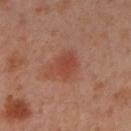Assessment: Part of a total-body skin-imaging series; this lesion was reviewed on a skin check and was not flagged for biopsy. Context: The patient is a female aged approximately 40. An algorithmic analysis of the crop reported a lesion area of about 7 mm², an outline eccentricity of about 0.6 (0 = round, 1 = elongated), and a shape-asymmetry score of about 0.25 (0 = symmetric). And it measured roughly 8 lightness units darker than nearby skin. The software also gave internal color variation of about 2 on a 0–10 scale and peripheral color asymmetry of about 0.5. The software also gave a nevus-likeness score of about 85/100 and a lesion-detection confidence of about 100/100. The lesion is on the arm. Approximately 3.5 mm at its widest. A 15 mm close-up tile from a total-body photography series done for melanoma screening. The tile uses cross-polarized illumination.